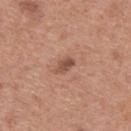Findings:
- follow-up — total-body-photography surveillance lesion; no biopsy
- lighting — white-light illumination
- imaging modality — 15 mm crop, total-body photography
- body site — the upper back
- subject — female, aged around 60
- diameter — about 2.5 mm